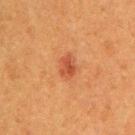The lesion was tiled from a total-body skin photograph and was not biopsied. Automated image analysis of the tile measured a lesion–skin lightness drop of about 9 and a normalized lesion–skin contrast near 7. And it measured a border-irregularity index near 2.5/10. A female patient aged 38 to 42. Located on the right upper arm. A 15 mm close-up tile from a total-body photography series done for melanoma screening.